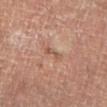An algorithmic analysis of the crop reported an automated nevus-likeness rating near 0 out of 100 and lesion-presence confidence of about 55/100. Located on the left lower leg. A close-up tile cropped from a whole-body skin photograph, about 15 mm across. The recorded lesion diameter is about 3 mm. The patient is a male approximately 70 years of age.